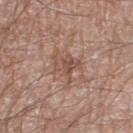This lesion was catalogued during total-body skin photography and was not selected for biopsy. An algorithmic analysis of the crop reported a lesion color around L≈51 a*≈19 b*≈27 in CIELAB and a normalized lesion–skin contrast near 6.5. Captured under white-light illumination. A male subject aged around 60. The lesion's longest dimension is about 4.5 mm. A 15 mm close-up tile from a total-body photography series done for melanoma screening. From the right thigh.